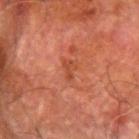Clinical impression:
No biopsy was performed on this lesion — it was imaged during a full skin examination and was not determined to be concerning.
Image and clinical context:
Captured under cross-polarized illumination. Approximately 2.5 mm at its widest. The subject is a male roughly 80 years of age. On the left forearm. A 15 mm close-up tile from a total-body photography series done for melanoma screening.